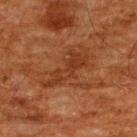Captured during whole-body skin photography for melanoma surveillance; the lesion was not biopsied.